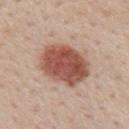Q: Is there a histopathology result?
A: no biopsy performed (imaged during a skin exam)